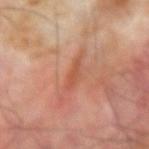Captured during whole-body skin photography for melanoma surveillance; the lesion was not biopsied.
A 15 mm close-up extracted from a 3D total-body photography capture.
The lesion is on the right forearm.
Measured at roughly 3 mm in maximum diameter.
A male subject, about 70 years old.
An algorithmic analysis of the crop reported about 7 CIELAB-L* units darker than the surrounding skin and a normalized lesion–skin contrast near 6. It also reported a border-irregularity rating of about 3.5/10, a within-lesion color-variation index near 0/10, and peripheral color asymmetry of about 0.
Imaged with cross-polarized lighting.